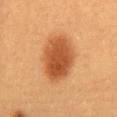Captured during whole-body skin photography for melanoma surveillance; the lesion was not biopsied.
A female subject approximately 30 years of age.
The lesion is on the abdomen.
The lesion-visualizer software estimated a lesion–skin lightness drop of about 14.
A region of skin cropped from a whole-body photographic capture, roughly 15 mm wide.
Measured at roughly 6.5 mm in maximum diameter.